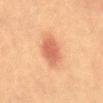The lesion was tiled from a total-body skin photograph and was not biopsied. Imaged with cross-polarized lighting. About 4.5 mm across. The lesion is on the mid back. The patient is a female aged 58 to 62. A 15 mm close-up extracted from a 3D total-body photography capture.